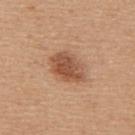Clinical impression:
The lesion was photographed on a routine skin check and not biopsied; there is no pathology result.
Acquisition and patient details:
An algorithmic analysis of the crop reported an outline eccentricity of about 0.75 (0 = round, 1 = elongated) and a shape-asymmetry score of about 0.15 (0 = symmetric). The analysis additionally found about 12 CIELAB-L* units darker than the surrounding skin and a lesion-to-skin contrast of about 8.5 (normalized; higher = more distinct). And it measured an automated nevus-likeness rating near 90 out of 100. A male subject, about 40 years old. The lesion is on the back. Cropped from a whole-body photographic skin survey; the tile spans about 15 mm. The tile uses white-light illumination. Approximately 4.5 mm at its widest.